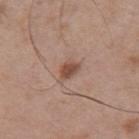Clinical impression:
Part of a total-body skin-imaging series; this lesion was reviewed on a skin check and was not flagged for biopsy.
Clinical summary:
On the back. A male subject, in their mid-50s. The tile uses white-light illumination. A 15 mm close-up tile from a total-body photography series done for melanoma screening.